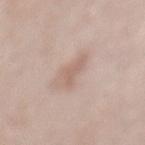biopsy_status: not biopsied; imaged during a skin examination